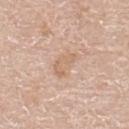Captured during whole-body skin photography for melanoma surveillance; the lesion was not biopsied. Automated image analysis of the tile measured an area of roughly 6 mm² and a symmetry-axis asymmetry near 0.3. The analysis additionally found a classifier nevus-likeness of about 0/100. The subject is a male aged 58–62. About 3.5 mm across. The lesion is located on the upper back. This is a white-light tile. Cropped from a total-body skin-imaging series; the visible field is about 15 mm.